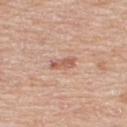Case summary:
- workup: no biopsy performed (imaged during a skin exam)
- lesion size: ≈3 mm
- anatomic site: the upper back
- subject: male, aged approximately 60
- imaging modality: ~15 mm tile from a whole-body skin photo